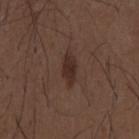{
  "lesion_size": {
    "long_diameter_mm_approx": 3.5
  },
  "patient": {
    "sex": "male",
    "age_approx": 50
  },
  "site": "mid back",
  "automated_metrics": {
    "cielab_L": 28,
    "cielab_a": 16,
    "cielab_b": 21,
    "vs_skin_darker_L": 8.0,
    "border_irregularity_0_10": 2.0,
    "color_variation_0_10": 1.5,
    "peripheral_color_asymmetry": 0.5
  },
  "image": {
    "source": "total-body photography crop",
    "field_of_view_mm": 15
  },
  "lighting": "white-light"
}